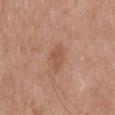The lesion was photographed on a routine skin check and not biopsied; there is no pathology result.
This is a white-light tile.
Measured at roughly 3 mm in maximum diameter.
The subject is a male aged approximately 55.
Cropped from a total-body skin-imaging series; the visible field is about 15 mm.
The total-body-photography lesion software estimated an area of roughly 4.5 mm² and two-axis asymmetry of about 0.25. And it measured a mean CIELAB color near L≈53 a*≈23 b*≈32 and about 7 CIELAB-L* units darker than the surrounding skin.
The lesion is on the upper back.